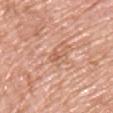Recorded during total-body skin imaging; not selected for excision or biopsy. Captured under white-light illumination. From the upper back. A roughly 15 mm field-of-view crop from a total-body skin photograph. The patient is a male aged 53 to 57.